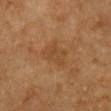Recorded during total-body skin imaging; not selected for excision or biopsy.